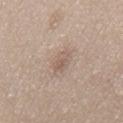Case summary:
- biopsy status: no biopsy performed (imaged during a skin exam)
- lesion diameter: about 3 mm
- site: the mid back
- lighting: white-light
- patient: male, aged 43–47
- automated metrics: a lesion color around L≈58 a*≈15 b*≈25 in CIELAB and a normalized lesion–skin contrast near 5.5; a nevus-likeness score of about 5/100 and lesion-presence confidence of about 100/100
- imaging modality: total-body-photography crop, ~15 mm field of view